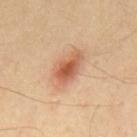<lesion>
<biopsy_status>not biopsied; imaged during a skin examination</biopsy_status>
<image>
  <source>total-body photography crop</source>
  <field_of_view_mm>15</field_of_view_mm>
</image>
<lighting>cross-polarized</lighting>
<patient>
  <sex>male</sex>
  <age_approx>55</age_approx>
</patient>
<lesion_size>
  <long_diameter_mm_approx>4.0</long_diameter_mm_approx>
</lesion_size>
<site>mid back</site>
</lesion>